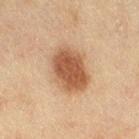biopsy status=total-body-photography surveillance lesion; no biopsy | lesion diameter=~5.5 mm (longest diameter) | image=total-body-photography crop, ~15 mm field of view | location=the right thigh | illumination=cross-polarized | automated metrics=a footprint of about 17 mm², an eccentricity of roughly 0.7, and two-axis asymmetry of about 0.15; a lesion-detection confidence of about 100/100 | patient=female, aged 53 to 57.